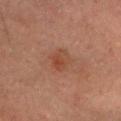| feature | finding |
|---|---|
| notes | imaged on a skin check; not biopsied |
| imaging modality | 15 mm crop, total-body photography |
| subject | male, about 80 years old |
| image-analysis metrics | a lesion color around L≈35 a*≈19 b*≈25 in CIELAB, about 5 CIELAB-L* units darker than the surrounding skin, and a normalized border contrast of about 5.5; a border-irregularity index near 2.5/10, internal color variation of about 6 on a 0–10 scale, and peripheral color asymmetry of about 2.5 |
| body site | the head or neck |
| lesion size | ~3.5 mm (longest diameter) |